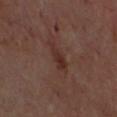Case summary:
* workup — total-body-photography surveillance lesion; no biopsy
* subject — in their mid- to late 60s
* imaging modality — ~15 mm tile from a whole-body skin photo
* body site — the head or neck
* illumination — cross-polarized illumination
* image-analysis metrics — a footprint of about 4 mm² and an outline eccentricity of about 0.85 (0 = round, 1 = elongated); an average lesion color of about L≈29 a*≈19 b*≈23 (CIELAB) and about 6 CIELAB-L* units darker than the surrounding skin; a classifier nevus-likeness of about 5/100 and a lesion-detection confidence of about 100/100